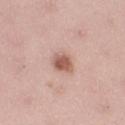Notes:
- notes · no biopsy performed (imaged during a skin exam)
- automated lesion analysis · a border-irregularity index near 1.5/10, a within-lesion color-variation index near 4/10, and peripheral color asymmetry of about 1.5
- acquisition · ~15 mm tile from a whole-body skin photo
- anatomic site · the left lower leg
- lesion diameter · ≈3 mm
- subject · female, in their mid-20s